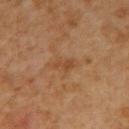Q: Was this lesion biopsied?
A: total-body-photography surveillance lesion; no biopsy
Q: Lesion location?
A: the mid back
Q: What is the imaging modality?
A: total-body-photography crop, ~15 mm field of view
Q: What is the lesion's diameter?
A: ≈2.5 mm
Q: Patient demographics?
A: male, about 60 years old
Q: Illumination type?
A: cross-polarized